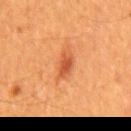follow-up = imaged on a skin check; not biopsied | diameter = ≈3 mm | body site = the back | patient = male, approximately 60 years of age | acquisition = ~15 mm crop, total-body skin-cancer survey | tile lighting = cross-polarized.